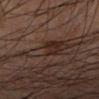Impression: Imaged during a routine full-body skin examination; the lesion was not biopsied and no histopathology is available. Context: The lesion-visualizer software estimated an average lesion color of about L≈28 a*≈15 b*≈21 (CIELAB), roughly 6 lightness units darker than nearby skin, and a normalized border contrast of about 7. It also reported border irregularity of about 4.5 on a 0–10 scale, internal color variation of about 7.5 on a 0–10 scale, and a peripheral color-asymmetry measure near 3. A male patient aged around 55. On the left forearm. Captured under cross-polarized illumination. A lesion tile, about 15 mm wide, cut from a 3D total-body photograph.